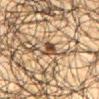Q: Was a biopsy performed?
A: catalogued during a skin exam; not biopsied
Q: How large is the lesion?
A: ~3 mm (longest diameter)
Q: What kind of image is this?
A: 15 mm crop, total-body photography
Q: Lesion location?
A: the mid back
Q: Patient demographics?
A: male, aged 63–67